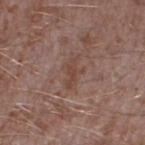| key | value |
|---|---|
| workup | total-body-photography surveillance lesion; no biopsy |
| image | ~15 mm crop, total-body skin-cancer survey |
| size | about 3.5 mm |
| subject | male, approximately 45 years of age |
| TBP lesion metrics | a lesion area of about 5 mm², an eccentricity of roughly 0.85, and two-axis asymmetry of about 0.45; a border-irregularity rating of about 6/10 and peripheral color asymmetry of about 0.5 |
| anatomic site | the right thigh |
| tile lighting | white-light |